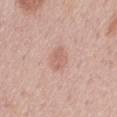Q: How was this image acquired?
A: ~15 mm tile from a whole-body skin photo
Q: What are the patient's age and sex?
A: male, approximately 70 years of age
Q: What is the anatomic site?
A: the back
Q: What lighting was used for the tile?
A: white-light illumination
Q: What did automated image analysis measure?
A: an area of roughly 2.5 mm², a shape eccentricity near 0.9, and two-axis asymmetry of about 0.45; an average lesion color of about L≈61 a*≈23 b*≈25 (CIELAB), roughly 8 lightness units darker than nearby skin, and a normalized border contrast of about 5.5; border irregularity of about 6 on a 0–10 scale and internal color variation of about 0 on a 0–10 scale; a classifier nevus-likeness of about 25/100 and a detector confidence of about 100 out of 100 that the crop contains a lesion